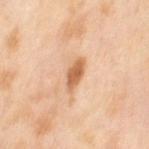Case summary:
– notes: imaged on a skin check; not biopsied
– patient: female, approximately 50 years of age
– tile lighting: cross-polarized illumination
– anatomic site: the left thigh
– lesion diameter: about 4 mm
– imaging modality: 15 mm crop, total-body photography
– image-analysis metrics: a normalized border contrast of about 9